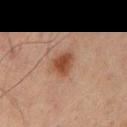The lesion is on the upper back. A male subject, aged 63 to 67. Captured under cross-polarized illumination. A lesion tile, about 15 mm wide, cut from a 3D total-body photograph.